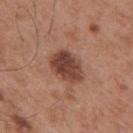notes=catalogued during a skin exam; not biopsied
image-analysis metrics=a border-irregularity rating of about 2/10, internal color variation of about 4 on a 0–10 scale, and a peripheral color-asymmetry measure near 1.5; an automated nevus-likeness rating near 50 out of 100 and a detector confidence of about 100 out of 100 that the crop contains a lesion
patient=male, in their mid-50s
image=15 mm crop, total-body photography
site=the mid back
lesion size=~4.5 mm (longest diameter)